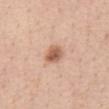{"biopsy_status": "not biopsied; imaged during a skin examination", "lighting": "white-light", "image": {"source": "total-body photography crop", "field_of_view_mm": 15}, "lesion_size": {"long_diameter_mm_approx": 2.5}, "patient": {"sex": "female", "age_approx": 55}, "site": "abdomen"}